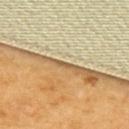follow-up: catalogued during a skin exam; not biopsied | patient: female, approximately 55 years of age | diameter: about 1.5 mm | location: the upper back | automated lesion analysis: a footprint of about 0.5 mm² and two-axis asymmetry of about 0.6 | image source: ~15 mm tile from a whole-body skin photo.